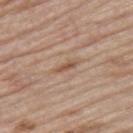Imaged during a routine full-body skin examination; the lesion was not biopsied and no histopathology is available.
The total-body-photography lesion software estimated a footprint of about 2.5 mm², an outline eccentricity of about 0.95 (0 = round, 1 = elongated), and two-axis asymmetry of about 0.2. And it measured a mean CIELAB color near L≈53 a*≈18 b*≈30 and roughly 9 lightness units darker than nearby skin. It also reported a classifier nevus-likeness of about 0/100 and a detector confidence of about 95 out of 100 that the crop contains a lesion.
Cropped from a whole-body photographic skin survey; the tile spans about 15 mm.
Imaged with white-light lighting.
The lesion is located on the right thigh.
A male patient roughly 70 years of age.